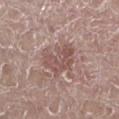Clinical impression: Imaged during a routine full-body skin examination; the lesion was not biopsied and no histopathology is available. Acquisition and patient details: A male subject aged approximately 65. A region of skin cropped from a whole-body photographic capture, roughly 15 mm wide. The lesion's longest dimension is about 4.5 mm. The lesion is on the leg.